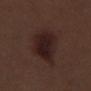follow-up — imaged on a skin check; not biopsied | anatomic site — the right lower leg | patient — male, approximately 70 years of age | image source — ~15 mm tile from a whole-body skin photo | illumination — white-light.